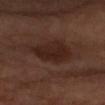The lesion was tiled from a total-body skin photograph and was not biopsied. The lesion is located on the arm. The subject is about 65 years old. A 15 mm close-up extracted from a 3D total-body photography capture.